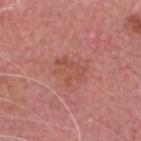Case summary:
- follow-up: imaged on a skin check; not biopsied
- image-analysis metrics: a border-irregularity index near 5/10, a color-variation rating of about 3/10, and radial color variation of about 1
- tile lighting: white-light
- size: ≈3.5 mm
- anatomic site: the head or neck
- subject: male, aged 73 to 77
- acquisition: total-body-photography crop, ~15 mm field of view